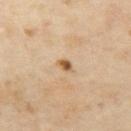Recorded during total-body skin imaging; not selected for excision or biopsy.
Cropped from a total-body skin-imaging series; the visible field is about 15 mm.
The subject is a female roughly 50 years of age.
Located on the left thigh.